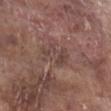notes: total-body-photography surveillance lesion; no biopsy | anatomic site: the right lower leg | patient: male, in their 70s | acquisition: ~15 mm tile from a whole-body skin photo | lesion size: about 4 mm | tile lighting: white-light illumination.